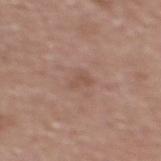Clinical impression: Imaged during a routine full-body skin examination; the lesion was not biopsied and no histopathology is available. Image and clinical context: A female patient, in their 70s. The lesion is located on the upper back. A close-up tile cropped from a whole-body skin photograph, about 15 mm across. About 2.5 mm across. This is a white-light tile.